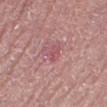Recorded during total-body skin imaging; not selected for excision or biopsy.
The subject is a female aged 63–67.
A close-up tile cropped from a whole-body skin photograph, about 15 mm across.
This is a white-light tile.
The lesion's longest dimension is about 2.5 mm.
From the right lower leg.